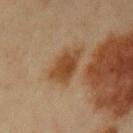Assessment: No biopsy was performed on this lesion — it was imaged during a full skin examination and was not determined to be concerning. Clinical summary: A 15 mm close-up extracted from a 3D total-body photography capture. A female patient approximately 45 years of age. Approximately 4 mm at its widest. Captured under cross-polarized illumination. From the left upper arm. The lesion-visualizer software estimated a lesion area of about 9.5 mm², a shape eccentricity near 0.7, and a shape-asymmetry score of about 0.3 (0 = symmetric). It also reported a border-irregularity index near 3/10, internal color variation of about 3 on a 0–10 scale, and peripheral color asymmetry of about 1. The software also gave a lesion-detection confidence of about 100/100.